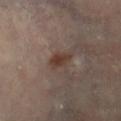* biopsy status · catalogued during a skin exam; not biopsied
* lesion diameter · ≈3 mm
* subject · female, approximately 60 years of age
* location · the right lower leg
* acquisition · ~15 mm tile from a whole-body skin photo